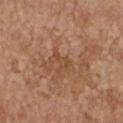Clinical impression: The lesion was photographed on a routine skin check and not biopsied; there is no pathology result. Background: Approximately 2.5 mm at its widest. The total-body-photography lesion software estimated a lesion area of about 3 mm². The analysis additionally found a lesion-detection confidence of about 90/100. A roughly 15 mm field-of-view crop from a total-body skin photograph. A female subject aged 63–67. The lesion is located on the chest.